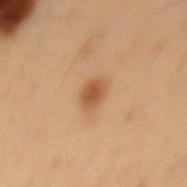Context: A close-up tile cropped from a whole-body skin photograph, about 15 mm across. The lesion is located on the mid back. The tile uses cross-polarized illumination. Approximately 3 mm at its widest. The subject is a male roughly 55 years of age.